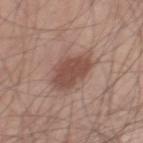Assessment:
Imaged during a routine full-body skin examination; the lesion was not biopsied and no histopathology is available.
Context:
A male subject in their mid-40s. The lesion-visualizer software estimated an area of roughly 14 mm², an eccentricity of roughly 0.7, and a symmetry-axis asymmetry near 0.2. And it measured border irregularity of about 2.5 on a 0–10 scale and a within-lesion color-variation index near 2.5/10. The lesion is located on the right thigh. A region of skin cropped from a whole-body photographic capture, roughly 15 mm wide. Measured at roughly 5 mm in maximum diameter. Captured under white-light illumination.